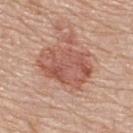Findings:
– workup · catalogued during a skin exam; not biopsied
– patient · male, aged 68 to 72
– lesion size · ~6.5 mm (longest diameter)
– location · the upper back
– automated lesion analysis · a lesion area of about 23 mm² and a shape eccentricity near 0.75; a border-irregularity index near 2/10, internal color variation of about 5.5 on a 0–10 scale, and peripheral color asymmetry of about 2; a nevus-likeness score of about 20/100 and lesion-presence confidence of about 100/100
– lighting · white-light
– acquisition · ~15 mm crop, total-body skin-cancer survey